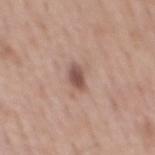notes = catalogued during a skin exam; not biopsied | subject = male, in their mid-50s | illumination = white-light illumination | diameter = ≈3 mm | automated lesion analysis = a lesion color around L≈52 a*≈19 b*≈24 in CIELAB and about 13 CIELAB-L* units darker than the surrounding skin; an automated nevus-likeness rating near 75 out of 100 and a detector confidence of about 100 out of 100 that the crop contains a lesion | image source = total-body-photography crop, ~15 mm field of view | site = the mid back.